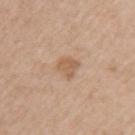The lesion is on the left upper arm. The total-body-photography lesion software estimated a footprint of about 5 mm², an outline eccentricity of about 0.45 (0 = round, 1 = elongated), and two-axis asymmetry of about 0.25. The software also gave a classifier nevus-likeness of about 0/100. The tile uses white-light illumination. Measured at roughly 2.5 mm in maximum diameter. A 15 mm crop from a total-body photograph taken for skin-cancer surveillance. The patient is a male about 60 years old.